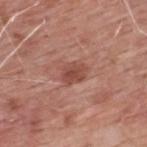{
  "image": {
    "source": "total-body photography crop",
    "field_of_view_mm": 15
  },
  "patient": {
    "sex": "male",
    "age_approx": 60
  },
  "lesion_size": {
    "long_diameter_mm_approx": 3.0
  },
  "site": "upper back"
}